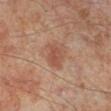Q: Is there a histopathology result?
A: no biopsy performed (imaged during a skin exam)
Q: What did automated image analysis measure?
A: a mean CIELAB color near L≈50 a*≈23 b*≈30, about 8 CIELAB-L* units darker than the surrounding skin, and a lesion-to-skin contrast of about 6.5 (normalized; higher = more distinct); border irregularity of about 2 on a 0–10 scale and a peripheral color-asymmetry measure near 1; a nevus-likeness score of about 10/100 and lesion-presence confidence of about 100/100
Q: Lesion size?
A: about 2.5 mm
Q: What kind of image is this?
A: 15 mm crop, total-body photography
Q: Who is the patient?
A: male, aged around 50
Q: Lesion location?
A: the left leg